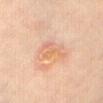Notes:
– notes · catalogued during a skin exam; not biopsied